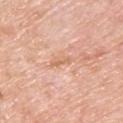No biopsy was performed on this lesion — it was imaged during a full skin examination and was not determined to be concerning.
Measured at roughly 3 mm in maximum diameter.
From the upper back.
The tile uses white-light illumination.
Cropped from a total-body skin-imaging series; the visible field is about 15 mm.
Automated tile analysis of the lesion measured a lesion area of about 3 mm², an outline eccentricity of about 0.95 (0 = round, 1 = elongated), and a shape-asymmetry score of about 0.3 (0 = symmetric). And it measured border irregularity of about 3.5 on a 0–10 scale, internal color variation of about 0 on a 0–10 scale, and radial color variation of about 0. The analysis additionally found a nevus-likeness score of about 0/100.
A male patient aged 78–82.